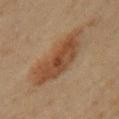Part of a total-body skin-imaging series; this lesion was reviewed on a skin check and was not flagged for biopsy.
About 8.5 mm across.
A lesion tile, about 15 mm wide, cut from a 3D total-body photograph.
Automated tile analysis of the lesion measured a border-irregularity rating of about 4/10, internal color variation of about 4 on a 0–10 scale, and radial color variation of about 1.
The lesion is located on the left upper arm.
A female subject, aged 43 to 47.